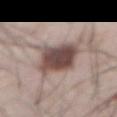| field | value |
|---|---|
| workup | total-body-photography surveillance lesion; no biopsy |
| illumination | white-light |
| automated metrics | a border-irregularity rating of about 2.5/10 and radial color variation of about 1.5 |
| size | ~6 mm (longest diameter) |
| patient | male, aged approximately 65 |
| imaging modality | total-body-photography crop, ~15 mm field of view |
| body site | the abdomen |